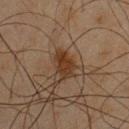Notes:
- TBP lesion metrics — a lesion area of about 4.5 mm², an eccentricity of roughly 0.75, and two-axis asymmetry of about 0.25; a lesion-detection confidence of about 100/100
- patient — male, approximately 45 years of age
- tile lighting — cross-polarized
- imaging modality — 15 mm crop, total-body photography
- diameter — ~2.5 mm (longest diameter)
- site — the chest